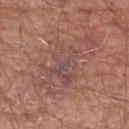Acquisition and patient details:
The lesion-visualizer software estimated an area of roughly 28 mm², an outline eccentricity of about 0.9 (0 = round, 1 = elongated), and two-axis asymmetry of about 0.45. The analysis additionally found a border-irregularity index near 8.5/10 and peripheral color asymmetry of about 1.5. The software also gave a classifier nevus-likeness of about 0/100 and lesion-presence confidence of about 80/100. From the right upper arm. This is a white-light tile. A 15 mm close-up tile from a total-body photography series done for melanoma screening. A male patient about 65 years old.